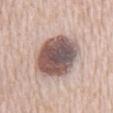follow-up: imaged on a skin check; not biopsied
subject: male, approximately 80 years of age
tile lighting: white-light illumination
diameter: about 7 mm
site: the chest
image-analysis metrics: a footprint of about 26 mm², an eccentricity of roughly 0.7, and a shape-asymmetry score of about 0.1 (0 = symmetric); a border-irregularity index near 1.5/10 and a color-variation rating of about 9/10; an automated nevus-likeness rating near 40 out of 100
imaging modality: ~15 mm crop, total-body skin-cancer survey